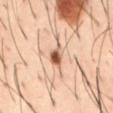<lesion>
  <biopsy_status>not biopsied; imaged during a skin examination</biopsy_status>
  <lighting>cross-polarized</lighting>
  <automated_metrics>
    <cielab_L>59</cielab_L>
    <cielab_a>21</cielab_a>
    <cielab_b>32</cielab_b>
    <vs_skin_contrast_norm>9.5</vs_skin_contrast_norm>
    <border_irregularity_0_10>3.0</border_irregularity_0_10>
    <peripheral_color_asymmetry>2.5</peripheral_color_asymmetry>
  </automated_metrics>
  <patient>
    <sex>male</sex>
    <age_approx>40</age_approx>
  </patient>
  <image>
    <source>total-body photography crop</source>
    <field_of_view_mm>15</field_of_view_mm>
  </image>
  <site>abdomen</site>
</lesion>